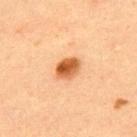This lesion was catalogued during total-body skin photography and was not selected for biopsy. The lesion is on the back. Cropped from a whole-body photographic skin survey; the tile spans about 15 mm. A male subject approximately 60 years of age. Measured at roughly 3 mm in maximum diameter.